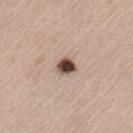Impression: Captured during whole-body skin photography for melanoma surveillance; the lesion was not biopsied. Context: The recorded lesion diameter is about 2.5 mm. This image is a 15 mm lesion crop taken from a total-body photograph. The lesion is on the left thigh. The tile uses white-light illumination. A male subject, aged 73 to 77.